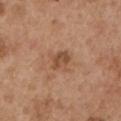biopsy status: no biopsy performed (imaged during a skin exam)
acquisition: total-body-photography crop, ~15 mm field of view
location: the left upper arm
patient: male, aged 53 to 57
diameter: ~2.5 mm (longest diameter)
illumination: white-light illumination
image-analysis metrics: a footprint of about 4 mm², a shape eccentricity near 0.7, and a shape-asymmetry score of about 0.2 (0 = symmetric); a border-irregularity rating of about 2/10, internal color variation of about 2 on a 0–10 scale, and peripheral color asymmetry of about 0.5; a lesion-detection confidence of about 100/100